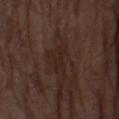site: leg
patient:
  sex: male
  age_approx: 75
image:
  source: total-body photography crop
  field_of_view_mm: 15